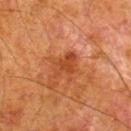Assessment: Recorded during total-body skin imaging; not selected for excision or biopsy. Image and clinical context: The patient is a male roughly 80 years of age. Approximately 3.5 mm at its widest. This is a cross-polarized tile. A region of skin cropped from a whole-body photographic capture, roughly 15 mm wide. The lesion-visualizer software estimated an average lesion color of about L≈36 a*≈25 b*≈33 (CIELAB), about 7 CIELAB-L* units darker than the surrounding skin, and a normalized lesion–skin contrast near 6.5. It also reported a border-irregularity rating of about 4/10 and peripheral color asymmetry of about 0.5. And it measured an automated nevus-likeness rating near 0 out of 100. From the leg.